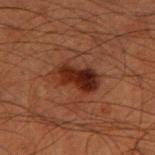Impression:
This lesion was catalogued during total-body skin photography and was not selected for biopsy.
Image and clinical context:
Approximately 5 mm at its widest. The lesion is located on the arm. The subject is a male aged 58 to 62. A roughly 15 mm field-of-view crop from a total-body skin photograph.